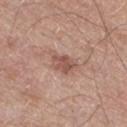| feature | finding |
|---|---|
| follow-up | no biopsy performed (imaged during a skin exam) |
| illumination | white-light illumination |
| size | ~2.5 mm (longest diameter) |
| subject | male, roughly 65 years of age |
| image source | 15 mm crop, total-body photography |
| automated lesion analysis | a nevus-likeness score of about 0/100 and a detector confidence of about 100 out of 100 that the crop contains a lesion |
| site | the leg |